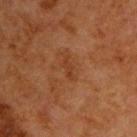Notes:
* follow-up · catalogued during a skin exam; not biopsied
* tile lighting · cross-polarized illumination
* automated metrics · a lesion color around L≈33 a*≈22 b*≈31 in CIELAB and a lesion–skin lightness drop of about 5; a border-irregularity index near 4.5/10 and internal color variation of about 0 on a 0–10 scale
* size · ~2.5 mm (longest diameter)
* subject · male, aged 63–67
* imaging modality · 15 mm crop, total-body photography
* body site · the upper back